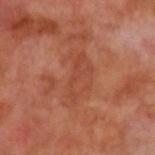| feature | finding |
|---|---|
| notes | imaged on a skin check; not biopsied |
| patient | male, aged approximately 70 |
| TBP lesion metrics | an eccentricity of roughly 0.85 and two-axis asymmetry of about 0.5; an automated nevus-likeness rating near 0 out of 100 and a lesion-detection confidence of about 100/100 |
| location | the left upper arm |
| acquisition | ~15 mm crop, total-body skin-cancer survey |
| lesion size | ≈5.5 mm |